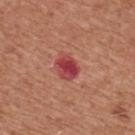Recorded during total-body skin imaging; not selected for excision or biopsy. A lesion tile, about 15 mm wide, cut from a 3D total-body photograph. The lesion's longest dimension is about 3 mm. The lesion is on the upper back. A male subject approximately 65 years of age.